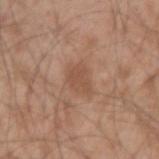Captured during whole-body skin photography for melanoma surveillance; the lesion was not biopsied.
About 3 mm across.
From the right forearm.
The subject is a male in their mid- to late 50s.
A 15 mm crop from a total-body photograph taken for skin-cancer surveillance.
The tile uses white-light illumination.